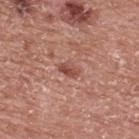Assessment:
No biopsy was performed on this lesion — it was imaged during a full skin examination and was not determined to be concerning.
Image and clinical context:
A region of skin cropped from a whole-body photographic capture, roughly 15 mm wide. A male patient aged 68–72. Captured under white-light illumination. Located on the upper back.